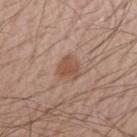<record>
  <biopsy_status>not biopsied; imaged during a skin examination</biopsy_status>
  <image>
    <source>total-body photography crop</source>
    <field_of_view_mm>15</field_of_view_mm>
  </image>
  <patient>
    <sex>male</sex>
    <age_approx>55</age_approx>
  </patient>
  <site>right forearm</site>
</record>